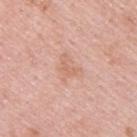Q: Was this lesion biopsied?
A: no biopsy performed (imaged during a skin exam)
Q: What is the anatomic site?
A: the back
Q: Illumination type?
A: white-light illumination
Q: What kind of image is this?
A: total-body-photography crop, ~15 mm field of view
Q: Who is the patient?
A: male, approximately 25 years of age
Q: What did automated image analysis measure?
A: an average lesion color of about L≈66 a*≈21 b*≈30 (CIELAB), roughly 6 lightness units darker than nearby skin, and a lesion-to-skin contrast of about 4.5 (normalized; higher = more distinct)
Q: What is the lesion's diameter?
A: ~3.5 mm (longest diameter)